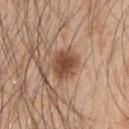The total-body-photography lesion software estimated an area of roughly 10 mm², an eccentricity of roughly 0.35, and a shape-asymmetry score of about 0.2 (0 = symmetric). The software also gave a border-irregularity rating of about 2/10 and a color-variation rating of about 3.5/10. And it measured an automated nevus-likeness rating near 100 out of 100 and lesion-presence confidence of about 100/100. The lesion's longest dimension is about 4 mm. The lesion is on the left upper arm. A male patient, approximately 50 years of age. Imaged with white-light lighting. A 15 mm crop from a total-body photograph taken for skin-cancer surveillance.